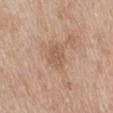The lesion was photographed on a routine skin check and not biopsied; there is no pathology result. A 15 mm crop from a total-body photograph taken for skin-cancer surveillance. From the mid back. The tile uses white-light illumination. A female subject roughly 60 years of age. An algorithmic analysis of the crop reported an eccentricity of roughly 0.5 and a symmetry-axis asymmetry near 0.25. The software also gave an average lesion color of about L≈57 a*≈19 b*≈31 (CIELAB), roughly 7 lightness units darker than nearby skin, and a normalized lesion–skin contrast near 5. The analysis additionally found a nevus-likeness score of about 0/100. Approximately 3 mm at its widest.